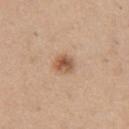The lesion was photographed on a routine skin check and not biopsied; there is no pathology result.
The recorded lesion diameter is about 2.5 mm.
On the chest.
A male subject approximately 40 years of age.
A 15 mm close-up extracted from a 3D total-body photography capture.
The total-body-photography lesion software estimated an area of roughly 5.5 mm², an eccentricity of roughly 0.55, and two-axis asymmetry of about 0.2. And it measured a normalized border contrast of about 8. The software also gave internal color variation of about 6 on a 0–10 scale and radial color variation of about 2. And it measured a classifier nevus-likeness of about 95/100.
Imaged with white-light lighting.